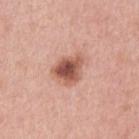Impression: Recorded during total-body skin imaging; not selected for excision or biopsy. Clinical summary: A male patient, aged 58–62. A 15 mm crop from a total-body photograph taken for skin-cancer surveillance. Located on the arm.